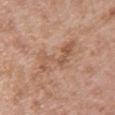Q: How was this image acquired?
A: 15 mm crop, total-body photography
Q: What is the anatomic site?
A: the back
Q: Illumination type?
A: white-light
Q: Who is the patient?
A: female, aged approximately 30
Q: Lesion size?
A: ~6 mm (longest diameter)